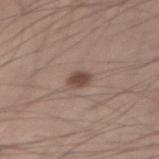Recorded during total-body skin imaging; not selected for excision or biopsy. The lesion is on the abdomen. The lesion-visualizer software estimated internal color variation of about 2 on a 0–10 scale and a peripheral color-asymmetry measure near 0.5. The lesion's longest dimension is about 2.5 mm. A close-up tile cropped from a whole-body skin photograph, about 15 mm across. A male subject in their mid-20s.